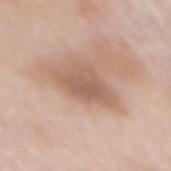{
  "biopsy_status": "not biopsied; imaged during a skin examination",
  "image": {
    "source": "total-body photography crop",
    "field_of_view_mm": 15
  },
  "automated_metrics": {
    "area_mm2_approx": 12.0,
    "eccentricity": 0.8,
    "vs_skin_darker_L": 10.0,
    "vs_skin_contrast_norm": 6.5,
    "border_irregularity_0_10": 3.5,
    "color_variation_0_10": 2.5
  },
  "lesion_size": {
    "long_diameter_mm_approx": 5.0
  },
  "patient": {
    "sex": "female",
    "age_approx": 60
  },
  "lighting": "white-light",
  "site": "mid back"
}